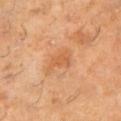The lesion was photographed on a routine skin check and not biopsied; there is no pathology result. Located on the right thigh. A male subject, about 60 years old. A close-up tile cropped from a whole-body skin photograph, about 15 mm across. Imaged with cross-polarized lighting. The recorded lesion diameter is about 3 mm.